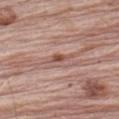{
  "biopsy_status": "not biopsied; imaged during a skin examination",
  "site": "arm",
  "image": {
    "source": "total-body photography crop",
    "field_of_view_mm": 15
  },
  "automated_metrics": {
    "border_irregularity_0_10": 5.0,
    "color_variation_0_10": 7.5
  },
  "lesion_size": {
    "long_diameter_mm_approx": 3.5
  },
  "patient": {
    "sex": "male",
    "age_approx": 65
  }
}